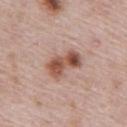follow-up: total-body-photography surveillance lesion; no biopsy
automated metrics: an area of roughly 10 mm², an eccentricity of roughly 0.85, and two-axis asymmetry of about 0.2; roughly 13 lightness units darker than nearby skin and a lesion-to-skin contrast of about 9.5 (normalized; higher = more distinct); a border-irregularity index near 3/10 and peripheral color asymmetry of about 2.5
site: the front of the torso
acquisition: 15 mm crop, total-body photography
lighting: white-light
subject: male, aged 68–72
lesion size: ~4.5 mm (longest diameter)